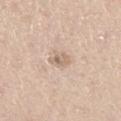Recorded during total-body skin imaging; not selected for excision or biopsy.
Located on the right thigh.
The lesion-visualizer software estimated a footprint of about 3 mm², an outline eccentricity of about 0.85 (0 = round, 1 = elongated), and a shape-asymmetry score of about 0.3 (0 = symmetric). The analysis additionally found about 10 CIELAB-L* units darker than the surrounding skin. It also reported internal color variation of about 4.5 on a 0–10 scale and peripheral color asymmetry of about 1.5. And it measured a classifier nevus-likeness of about 0/100 and a lesion-detection confidence of about 100/100.
A 15 mm close-up extracted from a 3D total-body photography capture.
A male patient aged 63–67.
This is a white-light tile.
The lesion's longest dimension is about 2.5 mm.